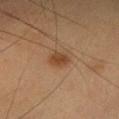Clinical impression: The lesion was photographed on a routine skin check and not biopsied; there is no pathology result. Context: A region of skin cropped from a whole-body photographic capture, roughly 15 mm wide. Located on the head or neck. A female subject aged 28–32.